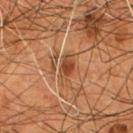image source: ~15 mm tile from a whole-body skin photo
body site: the chest
image-analysis metrics: a mean CIELAB color near L≈42 a*≈23 b*≈34 and roughly 10 lightness units darker than nearby skin; border irregularity of about 2.5 on a 0–10 scale and a color-variation rating of about 3.5/10; a classifier nevus-likeness of about 40/100 and a lesion-detection confidence of about 100/100
patient: male, in their mid- to late 50s
diameter: about 3 mm
illumination: cross-polarized illumination